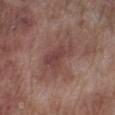The lesion was tiled from a total-body skin photograph and was not biopsied. A region of skin cropped from a whole-body photographic capture, roughly 15 mm wide. This is a white-light tile. A male patient aged 68–72. The lesion's longest dimension is about 4.5 mm. On the leg.